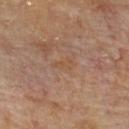Impression: Recorded during total-body skin imaging; not selected for excision or biopsy. Image and clinical context: From the upper back. A male subject, about 85 years old or older. This is a cross-polarized tile. A 15 mm close-up tile from a total-body photography series done for melanoma screening. Measured at roughly 3 mm in maximum diameter. The lesion-visualizer software estimated a footprint of about 3 mm², an outline eccentricity of about 0.9 (0 = round, 1 = elongated), and a symmetry-axis asymmetry near 0.35. The software also gave a border-irregularity rating of about 4/10 and internal color variation of about 0 on a 0–10 scale. The software also gave a nevus-likeness score of about 0/100 and a detector confidence of about 100 out of 100 that the crop contains a lesion.